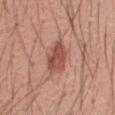Notes:
– illumination: white-light
– lesion size: about 4 mm
– automated lesion analysis: a within-lesion color-variation index near 4/10 and peripheral color asymmetry of about 1.5; a classifier nevus-likeness of about 85/100 and a lesion-detection confidence of about 100/100
– site: the mid back
– imaging modality: 15 mm crop, total-body photography
– subject: male, aged approximately 45